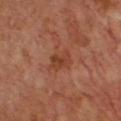A male subject, aged 63 to 67. A roughly 15 mm field-of-view crop from a total-body skin photograph. Automated image analysis of the tile measured about 7 CIELAB-L* units darker than the surrounding skin and a lesion-to-skin contrast of about 6.5 (normalized; higher = more distinct). The lesion's longest dimension is about 3 mm.